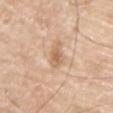The lesion was photographed on a routine skin check and not biopsied; there is no pathology result.